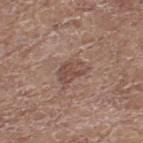<tbp_lesion>
  <biopsy_status>not biopsied; imaged during a skin examination</biopsy_status>
  <patient>
    <sex>female</sex>
    <age_approx>75</age_approx>
  </patient>
  <image>
    <source>total-body photography crop</source>
    <field_of_view_mm>15</field_of_view_mm>
  </image>
  <site>left thigh</site>
</tbp_lesion>